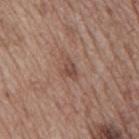Assessment:
Captured during whole-body skin photography for melanoma surveillance; the lesion was not biopsied.
Clinical summary:
On the mid back. A male subject aged approximately 70. The tile uses white-light illumination. A close-up tile cropped from a whole-body skin photograph, about 15 mm across.